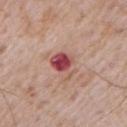This lesion was catalogued during total-body skin photography and was not selected for biopsy.